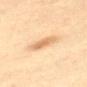Q: Was a biopsy performed?
A: total-body-photography surveillance lesion; no biopsy
Q: Patient demographics?
A: female, aged 63 to 67
Q: What is the imaging modality?
A: 15 mm crop, total-body photography
Q: Illumination type?
A: cross-polarized
Q: What is the anatomic site?
A: the mid back
Q: Automated lesion metrics?
A: a footprint of about 5.5 mm² and a symmetry-axis asymmetry near 0.2; a border-irregularity rating of about 2.5/10, internal color variation of about 3.5 on a 0–10 scale, and a peripheral color-asymmetry measure near 1; an automated nevus-likeness rating near 80 out of 100 and lesion-presence confidence of about 100/100
Q: What is the lesion's diameter?
A: ~3.5 mm (longest diameter)